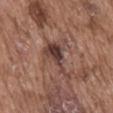Assessment: The lesion was photographed on a routine skin check and not biopsied; there is no pathology result. Acquisition and patient details: The lesion-visualizer software estimated an average lesion color of about L≈40 a*≈18 b*≈22 (CIELAB) and a lesion-to-skin contrast of about 9.5 (normalized; higher = more distinct). It also reported border irregularity of about 7.5 on a 0–10 scale. The analysis additionally found an automated nevus-likeness rating near 15 out of 100. Measured at roughly 5.5 mm in maximum diameter. The lesion is on the abdomen. A 15 mm close-up tile from a total-body photography series done for melanoma screening. The subject is a male aged 73–77.